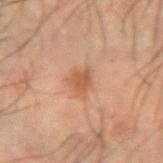Q: Was a biopsy performed?
A: imaged on a skin check; not biopsied
Q: Patient demographics?
A: male, about 60 years old
Q: How was the tile lit?
A: cross-polarized
Q: What is the imaging modality?
A: total-body-photography crop, ~15 mm field of view
Q: Where on the body is the lesion?
A: the arm
Q: What is the lesion's diameter?
A: ~3 mm (longest diameter)